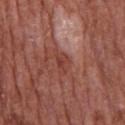{
  "biopsy_status": "not biopsied; imaged during a skin examination",
  "patient": {
    "sex": "male",
    "age_approx": 65
  },
  "lesion_size": {
    "long_diameter_mm_approx": 3.0
  },
  "image": {
    "source": "total-body photography crop",
    "field_of_view_mm": 15
  },
  "automated_metrics": {
    "border_irregularity_0_10": 4.0,
    "color_variation_0_10": 2.0,
    "nevus_likeness_0_100": 0,
    "lesion_detection_confidence_0_100": 80
  },
  "site": "chest"
}